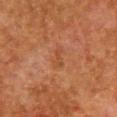notes=imaged on a skin check; not biopsied | tile lighting=cross-polarized | site=the chest | image source=total-body-photography crop, ~15 mm field of view | TBP lesion metrics=a color-variation rating of about 0/10; a classifier nevus-likeness of about 0/100 | size=~2.5 mm (longest diameter) | patient=female, approximately 50 years of age.